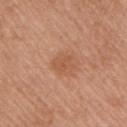• workup · catalogued during a skin exam; not biopsied
• tile lighting · white-light
• imaging modality · ~15 mm tile from a whole-body skin photo
• automated metrics · a classifier nevus-likeness of about 10/100 and a lesion-detection confidence of about 100/100
• subject · female, aged 58–62
• site · the arm
• size · ~2.5 mm (longest diameter)